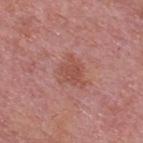biopsy status: total-body-photography surveillance lesion; no biopsy
diameter: ~3 mm (longest diameter)
image source: ~15 mm tile from a whole-body skin photo
patient: male, approximately 75 years of age
tile lighting: white-light illumination
site: the back
TBP lesion metrics: a border-irregularity index near 2/10, a within-lesion color-variation index near 1.5/10, and radial color variation of about 0.5